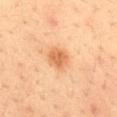Captured during whole-body skin photography for melanoma surveillance; the lesion was not biopsied. Automated tile analysis of the lesion measured a footprint of about 6 mm² and an outline eccentricity of about 0.4 (0 = round, 1 = elongated). The analysis additionally found a border-irregularity index near 1.5/10, a color-variation rating of about 2.5/10, and a peripheral color-asymmetry measure near 1. The analysis additionally found lesion-presence confidence of about 100/100. A male patient aged approximately 35. The lesion is located on the back. Approximately 3 mm at its widest. A 15 mm crop from a total-body photograph taken for skin-cancer surveillance.